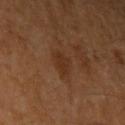Findings:
* follow-up · imaged on a skin check; not biopsied
* image · 15 mm crop, total-body photography
* patient · female, roughly 50 years of age
* image-analysis metrics · an area of roughly 5 mm², a shape eccentricity near 0.5, and a shape-asymmetry score of about 0.3 (0 = symmetric); a border-irregularity index near 3/10 and radial color variation of about 1; an automated nevus-likeness rating near 0 out of 100
* anatomic site · the left upper arm
* tile lighting · cross-polarized illumination
* lesion diameter · ≈2.5 mm